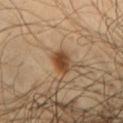Findings:
* workup: no biopsy performed (imaged during a skin exam)
* body site: the chest
* acquisition: ~15 mm crop, total-body skin-cancer survey